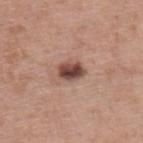Part of a total-body skin-imaging series; this lesion was reviewed on a skin check and was not flagged for biopsy. Located on the upper back. The subject is a male roughly 40 years of age. Approximately 3 mm at its widest. The total-body-photography lesion software estimated a mean CIELAB color near L≈46 a*≈20 b*≈24, roughly 16 lightness units darker than nearby skin, and a lesion-to-skin contrast of about 11.5 (normalized; higher = more distinct). A close-up tile cropped from a whole-body skin photograph, about 15 mm across.